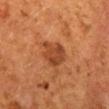Recorded during total-body skin imaging; not selected for excision or biopsy. The patient is a female aged around 50. Cropped from a whole-body photographic skin survey; the tile spans about 15 mm. Captured under cross-polarized illumination. The total-body-photography lesion software estimated an area of roughly 8.5 mm², an eccentricity of roughly 0.7, and a shape-asymmetry score of about 0.3 (0 = symmetric). And it measured a border-irregularity rating of about 3.5/10, a within-lesion color-variation index near 2/10, and radial color variation of about 0.5. And it measured an automated nevus-likeness rating near 65 out of 100 and lesion-presence confidence of about 100/100. From the mid back.